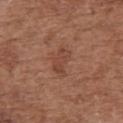Clinical impression:
No biopsy was performed on this lesion — it was imaged during a full skin examination and was not determined to be concerning.